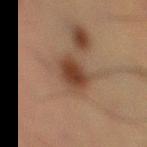Captured during whole-body skin photography for melanoma surveillance; the lesion was not biopsied.
Automated image analysis of the tile measured a lesion area of about 9.5 mm², an eccentricity of roughly 0.75, and a symmetry-axis asymmetry near 0.15. It also reported a lesion–skin lightness drop of about 10 and a normalized lesion–skin contrast near 9. The software also gave a border-irregularity rating of about 2/10 and a within-lesion color-variation index near 3/10.
Approximately 4.5 mm at its widest.
A 15 mm close-up tile from a total-body photography series done for melanoma screening.
From the leg.
Captured under cross-polarized illumination.
A male patient, aged approximately 55.